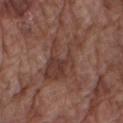Case summary:
– biopsy status: no biopsy performed (imaged during a skin exam)
– location: the right upper arm
– tile lighting: white-light
– size: ≈7 mm
– acquisition: total-body-photography crop, ~15 mm field of view
– subject: male, roughly 75 years of age